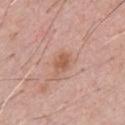| field | value |
|---|---|
| patient | male, roughly 50 years of age |
| imaging modality | ~15 mm tile from a whole-body skin photo |
| body site | the chest |
| diameter | about 2.5 mm |
| lighting | white-light illumination |
| automated metrics | roughly 9 lightness units darker than nearby skin and a normalized border contrast of about 7; a nevus-likeness score of about 65/100 |